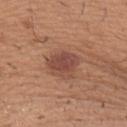Clinical impression: Imaged during a routine full-body skin examination; the lesion was not biopsied and no histopathology is available. Clinical summary: The subject is a male aged 38–42. On the right upper arm. Imaged with white-light lighting. A 15 mm close-up tile from a total-body photography series done for melanoma screening. The lesion's longest dimension is about 4 mm.